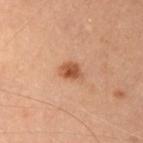No biopsy was performed on this lesion — it was imaged during a full skin examination and was not determined to be concerning. Longest diameter approximately 2.5 mm. Imaged with cross-polarized lighting. The lesion is on the back. A region of skin cropped from a whole-body photographic capture, roughly 15 mm wide. A female patient, aged 28 to 32.